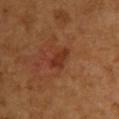{
  "biopsy_status": "not biopsied; imaged during a skin examination",
  "automated_metrics": {
    "cielab_L": 34,
    "cielab_a": 27,
    "cielab_b": 33,
    "vs_skin_darker_L": 8.0,
    "vs_skin_contrast_norm": 7.0,
    "nevus_likeness_0_100": 50,
    "lesion_detection_confidence_0_100": 100
  },
  "image": {
    "source": "total-body photography crop",
    "field_of_view_mm": 15
  },
  "lighting": "cross-polarized",
  "patient": {
    "sex": "female",
    "age_approx": 55
  },
  "lesion_size": {
    "long_diameter_mm_approx": 3.0
  },
  "site": "back"
}